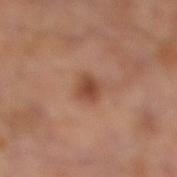The lesion was photographed on a routine skin check and not biopsied; there is no pathology result. On the leg. Approximately 2.5 mm at its widest. The subject is a male aged around 65. A roughly 15 mm field-of-view crop from a total-body skin photograph. Captured under cross-polarized illumination.